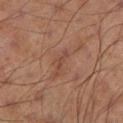Captured during whole-body skin photography for melanoma surveillance; the lesion was not biopsied.
The recorded lesion diameter is about 2.5 mm.
A male subject, aged approximately 70.
Automated tile analysis of the lesion measured a footprint of about 2 mm², a shape eccentricity near 0.95, and two-axis asymmetry of about 0.45.
The tile uses cross-polarized illumination.
A lesion tile, about 15 mm wide, cut from a 3D total-body photograph.
On the left lower leg.